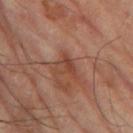{"biopsy_status": "not biopsied; imaged during a skin examination", "site": "leg", "lesion_size": {"long_diameter_mm_approx": 3.0}, "lighting": "cross-polarized", "automated_metrics": {"shape_asymmetry": 0.5, "cielab_L": 44, "cielab_a": 24, "cielab_b": 30, "vs_skin_contrast_norm": 6.5, "border_irregularity_0_10": 5.0, "color_variation_0_10": 5.0, "peripheral_color_asymmetry": 1.5, "nevus_likeness_0_100": 0}, "image": {"source": "total-body photography crop", "field_of_view_mm": 15}, "patient": {"sex": "male", "age_approx": 65}}